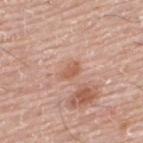The lesion was photographed on a routine skin check and not biopsied; there is no pathology result. About 2.5 mm across. The patient is a male aged 73–77. The lesion is located on the upper back. This image is a 15 mm lesion crop taken from a total-body photograph. Captured under white-light illumination.